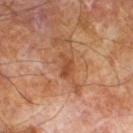| field | value |
|---|---|
| workup | no biopsy performed (imaged during a skin exam) |
| image | 15 mm crop, total-body photography |
| patient | male, aged 63 to 67 |
| lesion size | ≈2.5 mm |
| illumination | cross-polarized |